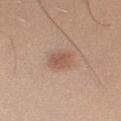Assessment: No biopsy was performed on this lesion — it was imaged during a full skin examination and was not determined to be concerning. Context: The total-body-photography lesion software estimated a lesion area of about 5.5 mm² and a shape eccentricity near 0.65. The analysis additionally found a lesion color around L≈55 a*≈20 b*≈28 in CIELAB, about 9 CIELAB-L* units darker than the surrounding skin, and a normalized lesion–skin contrast near 6.5. It also reported a classifier nevus-likeness of about 90/100 and lesion-presence confidence of about 100/100. The lesion is on the right upper arm. The patient is a male aged around 35. A roughly 15 mm field-of-view crop from a total-body skin photograph. The tile uses white-light illumination.